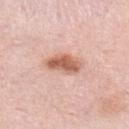Notes:
– follow-up — imaged on a skin check; not biopsied
– illumination — white-light
– patient — female, aged approximately 50
– image — ~15 mm tile from a whole-body skin photo
– TBP lesion metrics — a lesion color around L≈62 a*≈24 b*≈31 in CIELAB, roughly 14 lightness units darker than nearby skin, and a normalized lesion–skin contrast near 9; a lesion-detection confidence of about 100/100
– anatomic site — the abdomen
– lesion diameter — about 4.5 mm